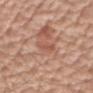biopsy status: total-body-photography surveillance lesion; no biopsy | image: ~15 mm crop, total-body skin-cancer survey | lesion diameter: ≈3.5 mm | subject: male, approximately 70 years of age | automated metrics: an outline eccentricity of about 0.9 (0 = round, 1 = elongated) and two-axis asymmetry of about 0.35; about 7 CIELAB-L* units darker than the surrounding skin and a normalized lesion–skin contrast near 5; a nevus-likeness score of about 20/100 and lesion-presence confidence of about 80/100 | lighting: white-light | body site: the right upper arm.